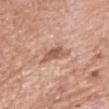Imaged during a routine full-body skin examination; the lesion was not biopsied and no histopathology is available.
About 3 mm across.
A female subject aged 73 to 77.
Imaged with white-light lighting.
A close-up tile cropped from a whole-body skin photograph, about 15 mm across.
The total-body-photography lesion software estimated a lesion color around L≈57 a*≈22 b*≈29 in CIELAB and a normalized border contrast of about 7.5. And it measured a nevus-likeness score of about 15/100 and a lesion-detection confidence of about 100/100.
Located on the right upper arm.